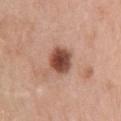No biopsy was performed on this lesion — it was imaged during a full skin examination and was not determined to be concerning.